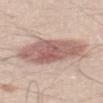No biopsy was performed on this lesion — it was imaged during a full skin examination and was not determined to be concerning. The patient is a male approximately 50 years of age. The tile uses white-light illumination. Automated image analysis of the tile measured a border-irregularity rating of about 2/10, internal color variation of about 4.5 on a 0–10 scale, and radial color variation of about 1.5. This image is a 15 mm lesion crop taken from a total-body photograph. Located on the mid back.